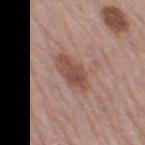On the right thigh. About 4.5 mm across. A roughly 15 mm field-of-view crop from a total-body skin photograph. The subject is a female in their mid-60s. Imaged with white-light lighting.